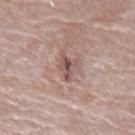Q: Was a biopsy performed?
A: no biopsy performed (imaged during a skin exam)
Q: How was this image acquired?
A: 15 mm crop, total-body photography
Q: What is the lesion's diameter?
A: about 2.5 mm
Q: What did automated image analysis measure?
A: a lesion area of about 4 mm², an eccentricity of roughly 0.6, and a shape-asymmetry score of about 0.45 (0 = symmetric); a lesion color around L≈51 a*≈18 b*≈21 in CIELAB, a lesion–skin lightness drop of about 11, and a lesion-to-skin contrast of about 7.5 (normalized; higher = more distinct); a color-variation rating of about 5/10; a lesion-detection confidence of about 100/100
Q: Illumination type?
A: white-light illumination
Q: Lesion location?
A: the arm
Q: Patient demographics?
A: female, about 65 years old